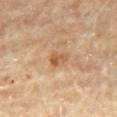Q: Is there a histopathology result?
A: no biopsy performed (imaged during a skin exam)
Q: Lesion location?
A: the mid back
Q: How was this image acquired?
A: total-body-photography crop, ~15 mm field of view
Q: What did automated image analysis measure?
A: an area of roughly 3.5 mm², a shape eccentricity near 0.75, and a symmetry-axis asymmetry near 0.4; a lesion color around L≈55 a*≈20 b*≈36 in CIELAB, about 9 CIELAB-L* units darker than the surrounding skin, and a normalized lesion–skin contrast near 7; internal color variation of about 3 on a 0–10 scale and peripheral color asymmetry of about 1; a classifier nevus-likeness of about 5/100
Q: Patient demographics?
A: male, roughly 85 years of age or older